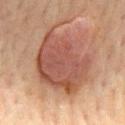Captured during whole-body skin photography for melanoma surveillance; the lesion was not biopsied. The lesion is on the abdomen. Automated tile analysis of the lesion measured a lesion area of about 39 mm² and two-axis asymmetry of about 0.2. The software also gave a border-irregularity rating of about 3/10, a within-lesion color-variation index near 4.5/10, and peripheral color asymmetry of about 1.5. The recorded lesion diameter is about 9 mm. A region of skin cropped from a whole-body photographic capture, roughly 15 mm wide. The subject is a male aged 68 to 72. Captured under cross-polarized illumination.